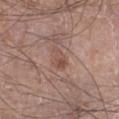Imaged during a routine full-body skin examination; the lesion was not biopsied and no histopathology is available. Located on the right lower leg. Automated image analysis of the tile measured a border-irregularity rating of about 3.5/10, a within-lesion color-variation index near 3.5/10, and a peripheral color-asymmetry measure near 1. A region of skin cropped from a whole-body photographic capture, roughly 15 mm wide. The tile uses white-light illumination. The recorded lesion diameter is about 3 mm. A male patient, in their mid-50s.